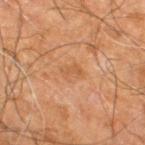Q: What did automated image analysis measure?
A: internal color variation of about 1.5 on a 0–10 scale and peripheral color asymmetry of about 0.5
Q: What are the patient's age and sex?
A: male, aged around 60
Q: What lighting was used for the tile?
A: cross-polarized
Q: What is the imaging modality?
A: total-body-photography crop, ~15 mm field of view
Q: How large is the lesion?
A: ~2.5 mm (longest diameter)
Q: Lesion location?
A: the right leg